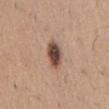biopsy_status: not biopsied; imaged during a skin examination
automated_metrics:
  cielab_L: 47
  cielab_a: 18
  cielab_b: 25
  vs_skin_darker_L: 18.0
  vs_skin_contrast_norm: 12.5
  border_irregularity_0_10: 1.5
  color_variation_0_10: 5.5
  peripheral_color_asymmetry: 1.5
image:
  source: total-body photography crop
  field_of_view_mm: 15
lighting: white-light
site: abdomen
patient:
  sex: male
  age_approx: 60
lesion_size:
  long_diameter_mm_approx: 4.0